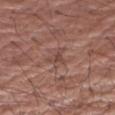Part of a total-body skin-imaging series; this lesion was reviewed on a skin check and was not flagged for biopsy.
About 2.5 mm across.
The subject is a male aged 68–72.
On the arm.
This is a white-light tile.
A region of skin cropped from a whole-body photographic capture, roughly 15 mm wide.